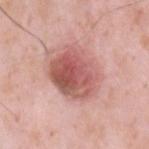Clinical impression: The lesion was photographed on a routine skin check and not biopsied; there is no pathology result. Clinical summary: Measured at roughly 6 mm in maximum diameter. A male patient, in their mid- to late 30s. This image is a 15 mm lesion crop taken from a total-body photograph. The lesion is on the upper back.